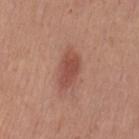This lesion was catalogued during total-body skin photography and was not selected for biopsy.
The lesion is located on the left thigh.
A female patient aged 38–42.
The lesion's longest dimension is about 4.5 mm.
A lesion tile, about 15 mm wide, cut from a 3D total-body photograph.
Imaged with white-light lighting.
Automated image analysis of the tile measured a mean CIELAB color near L≈49 a*≈25 b*≈28 and a lesion-to-skin contrast of about 7.5 (normalized; higher = more distinct).